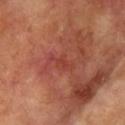Captured during whole-body skin photography for melanoma surveillance; the lesion was not biopsied.
The tile uses cross-polarized illumination.
Automated tile analysis of the lesion measured an area of roughly 4 mm², an outline eccentricity of about 0.9 (0 = round, 1 = elongated), and two-axis asymmetry of about 0.35. The software also gave a border-irregularity rating of about 4.5/10 and internal color variation of about 1 on a 0–10 scale. The analysis additionally found a nevus-likeness score of about 0/100 and a detector confidence of about 95 out of 100 that the crop contains a lesion.
From the right upper arm.
Approximately 3.5 mm at its widest.
A 15 mm close-up tile from a total-body photography series done for melanoma screening.
A female subject aged approximately 75.